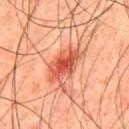Cropped from a whole-body photographic skin survey; the tile spans about 15 mm. A male patient in their mid- to late 40s. Located on the back. Captured under cross-polarized illumination. About 5 mm across. Automated tile analysis of the lesion measured an average lesion color of about L≈54 a*≈35 b*≈36 (CIELAB), a lesion–skin lightness drop of about 14, and a lesion-to-skin contrast of about 9 (normalized; higher = more distinct).